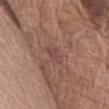Imaged during a routine full-body skin examination; the lesion was not biopsied and no histopathology is available. Cropped from a total-body skin-imaging series; the visible field is about 15 mm. From the left thigh. A female patient, roughly 75 years of age.